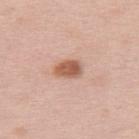{
  "biopsy_status": "not biopsied; imaged during a skin examination",
  "image": {
    "source": "total-body photography crop",
    "field_of_view_mm": 15
  },
  "site": "back",
  "lighting": "white-light",
  "lesion_size": {
    "long_diameter_mm_approx": 3.0
  },
  "patient": {
    "sex": "female",
    "age_approx": 65
  },
  "automated_metrics": {
    "vs_skin_contrast_norm": 9.5,
    "border_irregularity_0_10": 1.5,
    "color_variation_0_10": 3.0,
    "nevus_likeness_0_100": 95,
    "lesion_detection_confidence_0_100": 100
  }
}